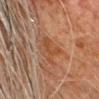Findings:
* notes · total-body-photography surveillance lesion; no biopsy
* image source · ~15 mm crop, total-body skin-cancer survey
* diameter · about 5.5 mm
* site · the head or neck
* subject · male, about 80 years old
* illumination · cross-polarized